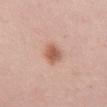The lesion was photographed on a routine skin check and not biopsied; there is no pathology result. Measured at roughly 4 mm in maximum diameter. A region of skin cropped from a whole-body photographic capture, roughly 15 mm wide. Located on the right thigh. A female patient in their 30s.